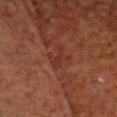<lesion>
  <biopsy_status>not biopsied; imaged during a skin examination</biopsy_status>
  <patient>
    <sex>male</sex>
    <age_approx>60</age_approx>
  </patient>
  <lesion_size>
    <long_diameter_mm_approx>3.5</long_diameter_mm_approx>
  </lesion_size>
  <site>head or neck</site>
  <image>
    <source>total-body photography crop</source>
    <field_of_view_mm>15</field_of_view_mm>
  </image>
</lesion>